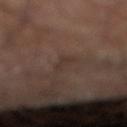The lesion was photographed on a routine skin check and not biopsied; there is no pathology result. Automated tile analysis of the lesion measured a mean CIELAB color near L≈33 a*≈15 b*≈21 and about 5 CIELAB-L* units darker than the surrounding skin. It also reported a border-irregularity index near 4/10 and a within-lesion color-variation index near 0/10. A 15 mm crop from a total-body photograph taken for skin-cancer surveillance. Measured at roughly 3 mm in maximum diameter. The lesion is located on the leg.